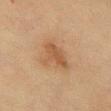<record>
<biopsy_status>not biopsied; imaged during a skin examination</biopsy_status>
<site>front of the torso</site>
<patient>
  <sex>female</sex>
  <age_approx>80</age_approx>
</patient>
<image>
  <source>total-body photography crop</source>
  <field_of_view_mm>15</field_of_view_mm>
</image>
<lesion_size>
  <long_diameter_mm_approx>4.5</long_diameter_mm_approx>
</lesion_size>
<lighting>cross-polarized</lighting>
<automated_metrics>
  <shape_asymmetry>0.4</shape_asymmetry>
</automated_metrics>
</record>